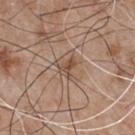The lesion was photographed on a routine skin check and not biopsied; there is no pathology result.
A region of skin cropped from a whole-body photographic capture, roughly 15 mm wide.
The patient is a male roughly 65 years of age.
Longest diameter approximately 3 mm.
Captured under white-light illumination.
Located on the chest.